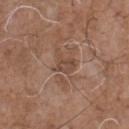Part of a total-body skin-imaging series; this lesion was reviewed on a skin check and was not flagged for biopsy.
The patient is a male roughly 70 years of age.
Imaged with white-light lighting.
A close-up tile cropped from a whole-body skin photograph, about 15 mm across.
About 2.5 mm across.
From the chest.